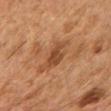Part of a total-body skin-imaging series; this lesion was reviewed on a skin check and was not flagged for biopsy. The lesion is located on the right upper arm. Cropped from a total-body skin-imaging series; the visible field is about 15 mm. A female subject aged around 60. Imaged with cross-polarized lighting.